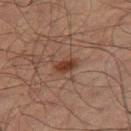Impression: This lesion was catalogued during total-body skin photography and was not selected for biopsy. Context: From the right thigh. The total-body-photography lesion software estimated a footprint of about 4 mm², an outline eccentricity of about 0.8 (0 = round, 1 = elongated), and two-axis asymmetry of about 0.25. This is a cross-polarized tile. A male patient, aged 48 to 52. This image is a 15 mm lesion crop taken from a total-body photograph.